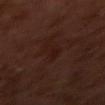The lesion was photographed on a routine skin check and not biopsied; there is no pathology result.
On the arm.
A male subject approximately 30 years of age.
A 15 mm close-up extracted from a 3D total-body photography capture.
An algorithmic analysis of the crop reported border irregularity of about 5.5 on a 0–10 scale, internal color variation of about 0.5 on a 0–10 scale, and a peripheral color-asymmetry measure near 0. The software also gave a nevus-likeness score of about 0/100.
The lesion's longest dimension is about 4 mm.